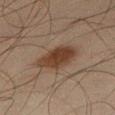biopsy_status: not biopsied; imaged during a skin examination
image:
  source: total-body photography crop
  field_of_view_mm: 15
site: right thigh
patient:
  sex: male
  age_approx: 45